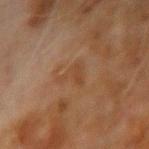Findings:
• notes · no biopsy performed (imaged during a skin exam)
• patient · male, aged 68–72
• imaging modality · 15 mm crop, total-body photography
• site · the right upper arm
• lesion diameter · ≈3.5 mm